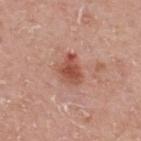Impression:
This lesion was catalogued during total-body skin photography and was not selected for biopsy.
Clinical summary:
A close-up tile cropped from a whole-body skin photograph, about 15 mm across. The patient is a male aged 73–77. From the mid back. Captured under white-light illumination. Measured at roughly 3.5 mm in maximum diameter.